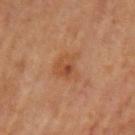Context: Captured under cross-polarized illumination. The lesion is on the arm. This image is a 15 mm lesion crop taken from a total-body photograph. A female subject in their mid- to late 60s.